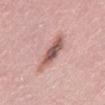Assessment: Part of a total-body skin-imaging series; this lesion was reviewed on a skin check and was not flagged for biopsy. Acquisition and patient details: A male subject, in their 30s. The recorded lesion diameter is about 5.5 mm. Imaged with white-light lighting. Automated tile analysis of the lesion measured a lesion color around L≈57 a*≈23 b*≈23 in CIELAB, a lesion–skin lightness drop of about 14, and a normalized lesion–skin contrast near 9. And it measured a nevus-likeness score of about 60/100. A 15 mm crop from a total-body photograph taken for skin-cancer surveillance. From the abdomen.